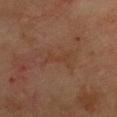The lesion was tiled from a total-body skin photograph and was not biopsied. The lesion is located on the chest. The lesion's longest dimension is about 3.5 mm. A female subject about 55 years old. A roughly 15 mm field-of-view crop from a total-body skin photograph. Imaged with cross-polarized lighting.